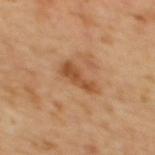biopsy status — catalogued during a skin exam; not biopsied | illumination — cross-polarized | imaging modality — ~15 mm crop, total-body skin-cancer survey | location — the mid back | diameter — ~4 mm (longest diameter) | patient — female, aged around 55 | TBP lesion metrics — a lesion area of about 6 mm² and an outline eccentricity of about 0.9 (0 = round, 1 = elongated); border irregularity of about 4.5 on a 0–10 scale and a within-lesion color-variation index near 2.5/10.